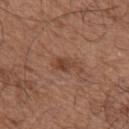| feature | finding |
|---|---|
| biopsy status | catalogued during a skin exam; not biopsied |
| tile lighting | white-light illumination |
| lesion diameter | ~3 mm (longest diameter) |
| site | the left upper arm |
| acquisition | ~15 mm crop, total-body skin-cancer survey |
| patient | male, aged approximately 50 |